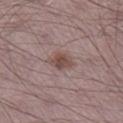<lesion>
<biopsy_status>not biopsied; imaged during a skin examination</biopsy_status>
<automated_metrics>
  <nevus_likeness_0_100>90</nevus_likeness_0_100>
  <lesion_detection_confidence_0_100>100</lesion_detection_confidence_0_100>
</automated_metrics>
<lighting>white-light</lighting>
<site>right lower leg</site>
<patient>
  <sex>male</sex>
  <age_approx>70</age_approx>
</patient>
<image>
  <source>total-body photography crop</source>
  <field_of_view_mm>15</field_of_view_mm>
</image>
</lesion>